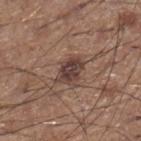No biopsy was performed on this lesion — it was imaged during a full skin examination and was not determined to be concerning. Located on the right lower leg. Cropped from a total-body skin-imaging series; the visible field is about 15 mm. A male subject about 60 years old.